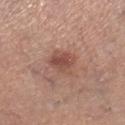Notes:
- workup — total-body-photography surveillance lesion; no biopsy
- site — the right lower leg
- subject — female, in their 30s
- image source — 15 mm crop, total-body photography
- automated metrics — a nevus-likeness score of about 55/100 and lesion-presence confidence of about 100/100
- lighting — white-light